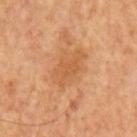This is a cross-polarized tile. A region of skin cropped from a whole-body photographic capture, roughly 15 mm wide. A male subject, aged 63 to 67. The lesion-visualizer software estimated an automated nevus-likeness rating near 0 out of 100 and a lesion-detection confidence of about 100/100. The recorded lesion diameter is about 5 mm.